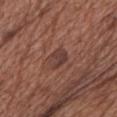lesion size=~3 mm (longest diameter); acquisition=total-body-photography crop, ~15 mm field of view; TBP lesion metrics=a border-irregularity rating of about 2/10, internal color variation of about 3 on a 0–10 scale, and peripheral color asymmetry of about 1; subject=female, aged 73–77; anatomic site=the chest; tile lighting=white-light illumination.